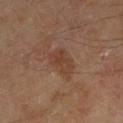biopsy status = catalogued during a skin exam; not biopsied | imaging modality = ~15 mm crop, total-body skin-cancer survey | tile lighting = cross-polarized illumination | TBP lesion metrics = an automated nevus-likeness rating near 0 out of 100 | diameter = ≈4 mm | subject = male, about 65 years old | site = the leg.